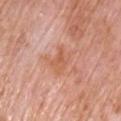biopsy status: catalogued during a skin exam; not biopsied | body site: the chest | patient: male, aged 58–62 | lighting: white-light | diameter: ~4.5 mm (longest diameter) | image source: ~15 mm tile from a whole-body skin photo.